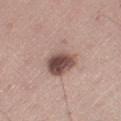Assessment: Imaged during a routine full-body skin examination; the lesion was not biopsied and no histopathology is available. Context: A male subject, aged 58–62. The lesion's longest dimension is about 5.5 mm. The tile uses white-light illumination. A roughly 15 mm field-of-view crop from a total-body skin photograph. Automated image analysis of the tile measured a classifier nevus-likeness of about 70/100 and a detector confidence of about 100 out of 100 that the crop contains a lesion. From the right thigh.